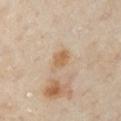Clinical impression: Imaged during a routine full-body skin examination; the lesion was not biopsied and no histopathology is available. Context: Automated image analysis of the tile measured a shape eccentricity near 0.8 and a shape-asymmetry score of about 0.25 (0 = symmetric). It also reported lesion-presence confidence of about 100/100. Captured under cross-polarized illumination. A 15 mm close-up tile from a total-body photography series done for melanoma screening. From the front of the torso. Longest diameter approximately 3.5 mm. A female subject, aged 58–62.